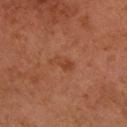Q: What kind of image is this?
A: ~15 mm crop, total-body skin-cancer survey
Q: Where on the body is the lesion?
A: the left upper arm
Q: Patient demographics?
A: female, aged approximately 60